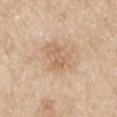Q: Was this lesion biopsied?
A: no biopsy performed (imaged during a skin exam)
Q: What is the imaging modality?
A: ~15 mm crop, total-body skin-cancer survey
Q: What lighting was used for the tile?
A: white-light
Q: Lesion size?
A: about 3 mm
Q: Patient demographics?
A: male, about 80 years old
Q: Lesion location?
A: the left upper arm